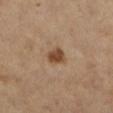The lesion was tiled from a total-body skin photograph and was not biopsied. The total-body-photography lesion software estimated an area of roughly 4.5 mm², an outline eccentricity of about 0.55 (0 = round, 1 = elongated), and a shape-asymmetry score of about 0.25 (0 = symmetric). And it measured a nevus-likeness score of about 95/100 and a detector confidence of about 100 out of 100 that the crop contains a lesion. The patient is a female aged 53–57. Captured under cross-polarized illumination. Cropped from a total-body skin-imaging series; the visible field is about 15 mm. On the left lower leg. Approximately 2.5 mm at its widest.